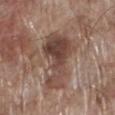Part of a total-body skin-imaging series; this lesion was reviewed on a skin check and was not flagged for biopsy.
A male subject approximately 70 years of age.
About 7.5 mm across.
An algorithmic analysis of the crop reported border irregularity of about 8 on a 0–10 scale, a color-variation rating of about 6/10, and a peripheral color-asymmetry measure near 2. It also reported lesion-presence confidence of about 100/100.
A lesion tile, about 15 mm wide, cut from a 3D total-body photograph.
The tile uses white-light illumination.
From the left lower leg.